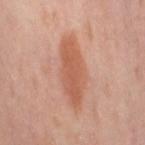biopsy_status: not biopsied; imaged during a skin examination
image:
  source: total-body photography crop
  field_of_view_mm: 15
lesion_size:
  long_diameter_mm_approx: 7.5
site: leg
lighting: cross-polarized
patient:
  sex: female
  age_approx: 50